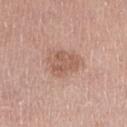The lesion was tiled from a total-body skin photograph and was not biopsied. A 15 mm close-up tile from a total-body photography series done for melanoma screening. The subject is a female aged approximately 65. On the right lower leg.